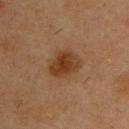The lesion was photographed on a routine skin check and not biopsied; there is no pathology result.
A roughly 15 mm field-of-view crop from a total-body skin photograph.
The lesion is on the front of the torso.
Longest diameter approximately 4 mm.
A male subject aged around 55.
Captured under cross-polarized illumination.